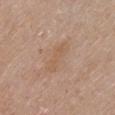notes: catalogued during a skin exam; not biopsied | lesion size: ~4.5 mm (longest diameter) | lighting: white-light | body site: the chest | automated metrics: an outline eccentricity of about 0.95 (0 = round, 1 = elongated) and a shape-asymmetry score of about 0.25 (0 = symmetric); a mean CIELAB color near L≈57 a*≈18 b*≈31 and a lesion-to-skin contrast of about 5 (normalized; higher = more distinct); a border-irregularity index near 4/10, a color-variation rating of about 1.5/10, and a peripheral color-asymmetry measure near 0.5 | image: ~15 mm tile from a whole-body skin photo | subject: female, in their mid-60s.